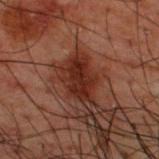Captured during whole-body skin photography for melanoma surveillance; the lesion was not biopsied. A lesion tile, about 15 mm wide, cut from a 3D total-body photograph. From the back. Captured under cross-polarized illumination. Approximately 4 mm at its widest. A male subject aged 48–52.